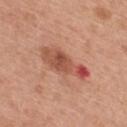Q: Was this lesion biopsied?
A: imaged on a skin check; not biopsied
Q: What is the imaging modality?
A: ~15 mm crop, total-body skin-cancer survey
Q: Lesion size?
A: ~6.5 mm (longest diameter)
Q: Automated lesion metrics?
A: a footprint of about 12 mm² and two-axis asymmetry of about 0.3; a lesion color around L≈52 a*≈28 b*≈31 in CIELAB, a lesion–skin lightness drop of about 12, and a normalized border contrast of about 8; a border-irregularity rating of about 4/10; an automated nevus-likeness rating near 0 out of 100
Q: Where on the body is the lesion?
A: the back
Q: Patient demographics?
A: male, aged 28 to 32
Q: What lighting was used for the tile?
A: white-light